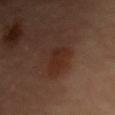Recorded during total-body skin imaging; not selected for excision or biopsy. The lesion's longest dimension is about 3.5 mm. A female subject aged 58–62. Located on the right arm. A roughly 15 mm field-of-view crop from a total-body skin photograph. The lesion-visualizer software estimated an area of roughly 6 mm², an outline eccentricity of about 0.75 (0 = round, 1 = elongated), and two-axis asymmetry of about 0.3. And it measured border irregularity of about 3 on a 0–10 scale and internal color variation of about 2.5 on a 0–10 scale.